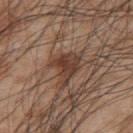Part of a total-body skin-imaging series; this lesion was reviewed on a skin check and was not flagged for biopsy.
The subject is a male in their mid- to late 40s.
Longest diameter approximately 5 mm.
The total-body-photography lesion software estimated a lesion color around L≈40 a*≈19 b*≈26 in CIELAB and a lesion–skin lightness drop of about 10.
A 15 mm close-up extracted from a 3D total-body photography capture.
Located on the upper back.